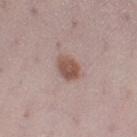biopsy status — no biopsy performed (imaged during a skin exam)
anatomic site — the left thigh
subject — female, in their 30s
image source — total-body-photography crop, ~15 mm field of view